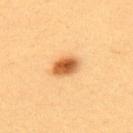  site: upper back
  image:
    source: total-body photography crop
    field_of_view_mm: 15
  patient:
    sex: female
    age_approx: 40
  lighting: cross-polarized